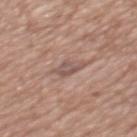Recorded during total-body skin imaging; not selected for excision or biopsy.
A male subject aged 63 to 67.
On the mid back.
A 15 mm crop from a total-body photograph taken for skin-cancer surveillance.
This is a white-light tile.
The recorded lesion diameter is about 3 mm.